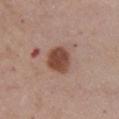Part of a total-body skin-imaging series; this lesion was reviewed on a skin check and was not flagged for biopsy.
The lesion's longest dimension is about 3.5 mm.
The lesion is on the front of the torso.
A female patient aged approximately 65.
A region of skin cropped from a whole-body photographic capture, roughly 15 mm wide.